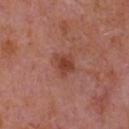biopsy_status: not biopsied; imaged during a skin examination
image:
  source: total-body photography crop
  field_of_view_mm: 15
patient:
  sex: male
  age_approx: 65
site: chest